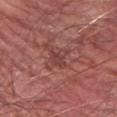Notes:
• workup — imaged on a skin check; not biopsied
• subject — male, aged 58 to 62
• lighting — white-light illumination
• acquisition — ~15 mm tile from a whole-body skin photo
• image-analysis metrics — an outline eccentricity of about 0.6 (0 = round, 1 = elongated); an average lesion color of about L≈42 a*≈24 b*≈24 (CIELAB)
• anatomic site — the right forearm